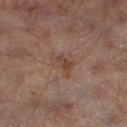Findings:
– notes: total-body-photography surveillance lesion; no biopsy
– image source: 15 mm crop, total-body photography
– lesion size: about 3.5 mm
– body site: the left lower leg
– tile lighting: cross-polarized illumination
– automated lesion analysis: an area of roughly 5.5 mm², an eccentricity of roughly 0.7, and a symmetry-axis asymmetry near 0.55; a lesion color around L≈42 a*≈17 b*≈26 in CIELAB, roughly 6 lightness units darker than nearby skin, and a normalized lesion–skin contrast near 6; a border-irregularity index near 6/10, a color-variation rating of about 4/10, and a peripheral color-asymmetry measure near 1.5; an automated nevus-likeness rating near 0 out of 100 and a detector confidence of about 100 out of 100 that the crop contains a lesion
– patient: male, approximately 65 years of age